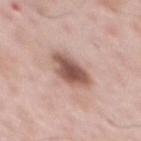Impression: Captured during whole-body skin photography for melanoma surveillance; the lesion was not biopsied. Acquisition and patient details: Located on the chest. A lesion tile, about 15 mm wide, cut from a 3D total-body photograph. A male subject aged around 60.